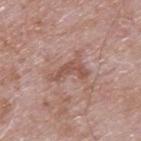Case summary:
• patient · male, about 65 years old
• site · the upper back
• illumination · white-light illumination
• lesion size · ~4.5 mm (longest diameter)
• image · 15 mm crop, total-body photography